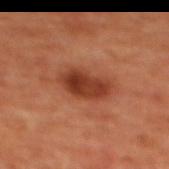Q: Was this lesion biopsied?
A: no biopsy performed (imaged during a skin exam)
Q: Lesion location?
A: the back
Q: What are the patient's age and sex?
A: female
Q: What is the imaging modality?
A: ~15 mm crop, total-body skin-cancer survey